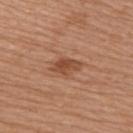This lesion was catalogued during total-body skin photography and was not selected for biopsy. On the upper back. Automated tile analysis of the lesion measured two-axis asymmetry of about 0.2. It also reported border irregularity of about 2.5 on a 0–10 scale, a color-variation rating of about 3/10, and a peripheral color-asymmetry measure near 1. The software also gave a nevus-likeness score of about 80/100. The recorded lesion diameter is about 3.5 mm. This is a white-light tile. A female patient, roughly 60 years of age. Cropped from a whole-body photographic skin survey; the tile spans about 15 mm.